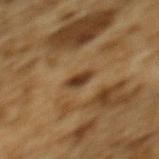Clinical impression: This lesion was catalogued during total-body skin photography and was not selected for biopsy. Background: The lesion is located on the mid back. A male subject, aged approximately 85 or older. A 15 mm close-up tile from a total-body photography series done for melanoma screening. An algorithmic analysis of the crop reported a border-irregularity index near 2/10, a within-lesion color-variation index near 3/10, and peripheral color asymmetry of about 1.